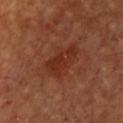Q: Was this lesion biopsied?
A: total-body-photography surveillance lesion; no biopsy
Q: Lesion location?
A: the chest
Q: What is the imaging modality?
A: total-body-photography crop, ~15 mm field of view
Q: Patient demographics?
A: male, approximately 50 years of age
Q: Automated lesion metrics?
A: a footprint of about 8 mm², an eccentricity of roughly 0.9, and a symmetry-axis asymmetry near 0.4; a lesion color around L≈31 a*≈28 b*≈31 in CIELAB, a lesion–skin lightness drop of about 7, and a lesion-to-skin contrast of about 7 (normalized; higher = more distinct); a border-irregularity rating of about 4.5/10, a within-lesion color-variation index near 2.5/10, and peripheral color asymmetry of about 1; a detector confidence of about 100 out of 100 that the crop contains a lesion
Q: What lighting was used for the tile?
A: cross-polarized